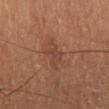Notes:
- workup · no biopsy performed (imaged during a skin exam)
- image-analysis metrics · an area of roughly 4.5 mm², an outline eccentricity of about 0.65 (0 = round, 1 = elongated), and a shape-asymmetry score of about 0.3 (0 = symmetric); border irregularity of about 3.5 on a 0–10 scale, internal color variation of about 1.5 on a 0–10 scale, and peripheral color asymmetry of about 0.5; lesion-presence confidence of about 100/100
- diameter · about 3 mm
- site · the leg
- patient · male, roughly 50 years of age
- imaging modality · ~15 mm crop, total-body skin-cancer survey
- lighting · cross-polarized illumination